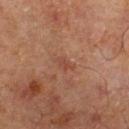Assessment: This lesion was catalogued during total-body skin photography and was not selected for biopsy. Background: The total-body-photography lesion software estimated an average lesion color of about L≈36 a*≈19 b*≈24 (CIELAB), roughly 5 lightness units darker than nearby skin, and a normalized lesion–skin contrast near 4.5. It also reported radial color variation of about 0.5. The lesion is on the left lower leg. Longest diameter approximately 2.5 mm. Captured under cross-polarized illumination. Cropped from a whole-body photographic skin survey; the tile spans about 15 mm. The patient is a male aged approximately 65.